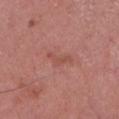biopsy_status: not biopsied; imaged during a skin examination
site: left lower leg
image:
  source: total-body photography crop
  field_of_view_mm: 15
lesion_size:
  long_diameter_mm_approx: 3.5
lighting: white-light
patient:
  sex: male
  age_approx: 85+